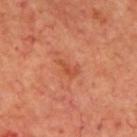Assessment: This lesion was catalogued during total-body skin photography and was not selected for biopsy. Image and clinical context: On the upper back. The tile uses cross-polarized illumination. A 15 mm close-up tile from a total-body photography series done for melanoma screening. The subject is a male aged approximately 70.